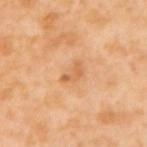Q: Was a biopsy performed?
A: catalogued during a skin exam; not biopsied
Q: How large is the lesion?
A: ≈3 mm
Q: How was this image acquired?
A: ~15 mm crop, total-body skin-cancer survey
Q: How was the tile lit?
A: cross-polarized illumination
Q: Patient demographics?
A: female, aged 53 to 57
Q: What is the anatomic site?
A: the upper back